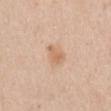No biopsy was performed on this lesion — it was imaged during a full skin examination and was not determined to be concerning. The lesion is located on the mid back. The recorded lesion diameter is about 3 mm. The tile uses white-light illumination. The total-body-photography lesion software estimated a footprint of about 4.5 mm², a shape eccentricity near 0.7, and two-axis asymmetry of about 0.3. The analysis additionally found a nevus-likeness score of about 45/100 and a detector confidence of about 100 out of 100 that the crop contains a lesion. The subject is a male aged around 60. A 15 mm crop from a total-body photograph taken for skin-cancer surveillance.